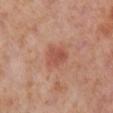Assessment:
This lesion was catalogued during total-body skin photography and was not selected for biopsy.
Clinical summary:
The lesion is located on the right lower leg. This is a cross-polarized tile. About 3 mm across. Cropped from a total-body skin-imaging series; the visible field is about 15 mm. A female subject aged around 55.